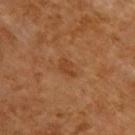Case summary:
– biopsy status: total-body-photography surveillance lesion; no biopsy
– anatomic site: the back
– tile lighting: cross-polarized
– acquisition: ~15 mm tile from a whole-body skin photo
– lesion diameter: ≈2.5 mm
– subject: male, about 60 years old
– automated lesion analysis: an area of roughly 3 mm², an outline eccentricity of about 0.8 (0 = round, 1 = elongated), and two-axis asymmetry of about 0.35; a border-irregularity rating of about 3.5/10, internal color variation of about 1 on a 0–10 scale, and radial color variation of about 0.5; a nevus-likeness score of about 0/100 and a lesion-detection confidence of about 100/100